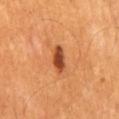The lesion was photographed on a routine skin check and not biopsied; there is no pathology result. Captured under cross-polarized illumination. Longest diameter approximately 3.5 mm. A close-up tile cropped from a whole-body skin photograph, about 15 mm across. From the mid back. A male subject aged 53 to 57.